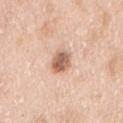Clinical summary:
A region of skin cropped from a whole-body photographic capture, roughly 15 mm wide. The subject is a female aged approximately 50. The lesion is on the right upper arm. About 3 mm across.